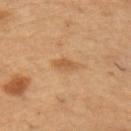A 15 mm close-up tile from a total-body photography series done for melanoma screening. Captured under cross-polarized illumination. The patient is a male aged around 55. An algorithmic analysis of the crop reported a lesion–skin lightness drop of about 8 and a lesion-to-skin contrast of about 6 (normalized; higher = more distinct). The analysis additionally found a within-lesion color-variation index near 1.5/10 and a peripheral color-asymmetry measure near 0.5. It also reported a detector confidence of about 100 out of 100 that the crop contains a lesion. From the right upper arm.